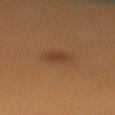Assessment: The lesion was photographed on a routine skin check and not biopsied; there is no pathology result. Image and clinical context: Located on the left lower leg. The patient is a female aged approximately 40. Measured at roughly 2.5 mm in maximum diameter. A region of skin cropped from a whole-body photographic capture, roughly 15 mm wide.